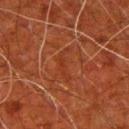The lesion was photographed on a routine skin check and not biopsied; there is no pathology result. The recorded lesion diameter is about 4.5 mm. The lesion is located on the arm. A male subject, approximately 80 years of age. A lesion tile, about 15 mm wide, cut from a 3D total-body photograph. This is a cross-polarized tile.